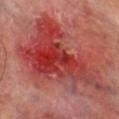biopsy status: total-body-photography surveillance lesion; no biopsy | patient: male, about 70 years old | image source: ~15 mm tile from a whole-body skin photo | anatomic site: the leg.